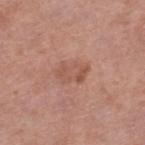Assessment: Part of a total-body skin-imaging series; this lesion was reviewed on a skin check and was not flagged for biopsy. Context: Measured at roughly 4 mm in maximum diameter. An algorithmic analysis of the crop reported a lesion area of about 7.5 mm², an outline eccentricity of about 0.75 (0 = round, 1 = elongated), and a shape-asymmetry score of about 0.25 (0 = symmetric). And it measured a lesion-to-skin contrast of about 5.5 (normalized; higher = more distinct). And it measured border irregularity of about 3 on a 0–10 scale, a color-variation rating of about 3/10, and a peripheral color-asymmetry measure near 1. And it measured an automated nevus-likeness rating near 0 out of 100 and a lesion-detection confidence of about 100/100. Imaged with white-light lighting. Cropped from a whole-body photographic skin survey; the tile spans about 15 mm. A female patient, aged approximately 55. The lesion is on the right lower leg.